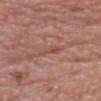Captured during whole-body skin photography for melanoma surveillance; the lesion was not biopsied. A roughly 15 mm field-of-view crop from a total-body skin photograph. This is a white-light tile. The subject is a male aged 53–57. Approximately 3.5 mm at its widest.